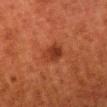Impression: Recorded during total-body skin imaging; not selected for excision or biopsy. Clinical summary: Imaged with cross-polarized lighting. A 15 mm close-up tile from a total-body photography series done for melanoma screening. A male patient aged around 80. Automated image analysis of the tile measured an average lesion color of about L≈29 a*≈25 b*≈29 (CIELAB), a lesion–skin lightness drop of about 8, and a normalized lesion–skin contrast near 7.5. It also reported a border-irregularity rating of about 2/10 and peripheral color asymmetry of about 1.5. The analysis additionally found a lesion-detection confidence of about 100/100. The lesion is located on the leg. Longest diameter approximately 3 mm.